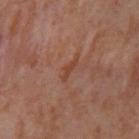Part of a total-body skin-imaging series; this lesion was reviewed on a skin check and was not flagged for biopsy. The lesion is located on the right upper arm. A female subject, about 50 years old. A 15 mm close-up tile from a total-body photography series done for melanoma screening.